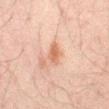Assessment:
The lesion was photographed on a routine skin check and not biopsied; there is no pathology result.
Context:
The tile uses cross-polarized illumination. An algorithmic analysis of the crop reported an average lesion color of about L≈53 a*≈20 b*≈29 (CIELAB) and a normalized border contrast of about 7. The software also gave a border-irregularity rating of about 2.5/10, internal color variation of about 2 on a 0–10 scale, and peripheral color asymmetry of about 1. A male subject, approximately 30 years of age. Located on the abdomen. A lesion tile, about 15 mm wide, cut from a 3D total-body photograph. The lesion's longest dimension is about 2.5 mm.